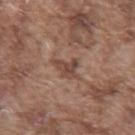{"biopsy_status": "not biopsied; imaged during a skin examination", "site": "chest", "patient": {"sex": "male", "age_approx": 75}, "automated_metrics": {"area_mm2_approx": 5.0, "shape_asymmetry": 0.5, "border_irregularity_0_10": 5.5, "color_variation_0_10": 2.0, "peripheral_color_asymmetry": 0.5, "nevus_likeness_0_100": 0, "lesion_detection_confidence_0_100": 90}, "lighting": "white-light", "image": {"source": "total-body photography crop", "field_of_view_mm": 15}, "lesion_size": {"long_diameter_mm_approx": 3.0}}